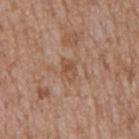* biopsy status · catalogued during a skin exam; not biopsied
* location · the mid back
* diameter · ~2.5 mm (longest diameter)
* tile lighting · white-light
* subject · male, aged around 65
* imaging modality · total-body-photography crop, ~15 mm field of view